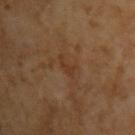Background: Captured under cross-polarized illumination. The recorded lesion diameter is about 2.5 mm. Cropped from a total-body skin-imaging series; the visible field is about 15 mm. A male subject about 65 years old.